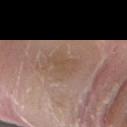This lesion was catalogued during total-body skin photography and was not selected for biopsy. A male patient, aged 38–42. The recorded lesion diameter is about 4 mm. The lesion is on the arm. Cropped from a total-body skin-imaging series; the visible field is about 15 mm.